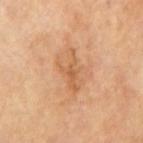biopsy status — no biopsy performed (imaged during a skin exam); location — the mid back; image — 15 mm crop, total-body photography; subject — male, in their 70s.